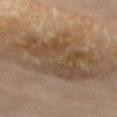The lesion was photographed on a routine skin check and not biopsied; there is no pathology result. The tile uses cross-polarized illumination. Automated image analysis of the tile measured a nevus-likeness score of about 0/100 and a detector confidence of about 95 out of 100 that the crop contains a lesion. A female patient, aged approximately 75. On the chest. Cropped from a total-body skin-imaging series; the visible field is about 15 mm.